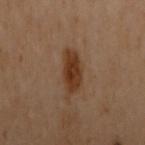biopsy status = total-body-photography surveillance lesion; no biopsy
subject = female, aged 58 to 62
location = the left arm
tile lighting = cross-polarized
imaging modality = 15 mm crop, total-body photography